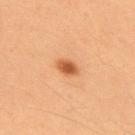Clinical impression: The lesion was tiled from a total-body skin photograph and was not biopsied. Context: Located on the upper back. About 3 mm across. This is a cross-polarized tile. A 15 mm close-up tile from a total-body photography series done for melanoma screening. A male subject in their mid-50s. Automated image analysis of the tile measured a lesion area of about 4 mm², a shape eccentricity near 0.75, and two-axis asymmetry of about 0.25. And it measured radial color variation of about 1.